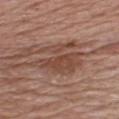Clinical impression: Imaged during a routine full-body skin examination; the lesion was not biopsied and no histopathology is available. Image and clinical context: The subject is a male aged 63–67. Longest diameter approximately 7 mm. A close-up tile cropped from a whole-body skin photograph, about 15 mm across. This is a white-light tile. The lesion is located on the chest.